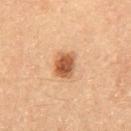Q: Is there a histopathology result?
A: imaged on a skin check; not biopsied
Q: What is the lesion's diameter?
A: ≈3 mm
Q: Patient demographics?
A: male, aged 58–62
Q: What lighting was used for the tile?
A: cross-polarized
Q: What is the imaging modality?
A: ~15 mm crop, total-body skin-cancer survey